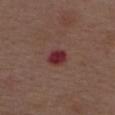Assessment: Part of a total-body skin-imaging series; this lesion was reviewed on a skin check and was not flagged for biopsy. Background: The patient is a male aged 68–72. Approximately 2.5 mm at its widest. From the upper back. Automated tile analysis of the lesion measured a mean CIELAB color near L≈32 a*≈28 b*≈20, a lesion–skin lightness drop of about 11, and a lesion-to-skin contrast of about 10.5 (normalized; higher = more distinct). And it measured an automated nevus-likeness rating near 0 out of 100 and a lesion-detection confidence of about 100/100. A close-up tile cropped from a whole-body skin photograph, about 15 mm across.